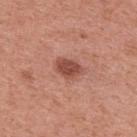notes: total-body-photography surveillance lesion; no biopsy
image: 15 mm crop, total-body photography
TBP lesion metrics: an outline eccentricity of about 0.75 (0 = round, 1 = elongated); a lesion color around L≈49 a*≈26 b*≈28 in CIELAB, a lesion–skin lightness drop of about 12, and a normalized lesion–skin contrast near 8.5; a border-irregularity rating of about 2/10, a color-variation rating of about 2.5/10, and a peripheral color-asymmetry measure near 1; a nevus-likeness score of about 85/100 and lesion-presence confidence of about 100/100
location: the upper back
illumination: white-light
patient: male, aged 53–57